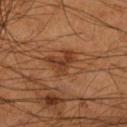  biopsy_status: not biopsied; imaged during a skin examination
  site: right lower leg
  image:
    source: total-body photography crop
    field_of_view_mm: 15
  patient:
    sex: male
    age_approx: 55
  automated_metrics:
    area_mm2_approx: 9.5
    eccentricity: 0.6
    shape_asymmetry: 0.4
    cielab_L: 36
    cielab_a: 21
    cielab_b: 31
    vs_skin_darker_L: 7.0
    peripheral_color_asymmetry: 1.5